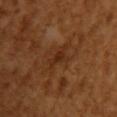<case>
  <biopsy_status>not biopsied; imaged during a skin examination</biopsy_status>
  <lesion_size>
    <long_diameter_mm_approx>3.0</long_diameter_mm_approx>
  </lesion_size>
  <site>arm</site>
  <patient>
    <sex>female</sex>
    <age_approx>55</age_approx>
  </patient>
  <lighting>cross-polarized</lighting>
  <image>
    <source>total-body photography crop</source>
    <field_of_view_mm>15</field_of_view_mm>
  </image>
</case>